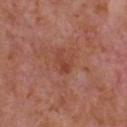follow-up = total-body-photography surveillance lesion; no biopsy
body site = the front of the torso
patient = male, in their mid-60s
image = 15 mm crop, total-body photography